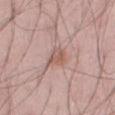Clinical impression:
Imaged during a routine full-body skin examination; the lesion was not biopsied and no histopathology is available.
Image and clinical context:
Measured at roughly 2.5 mm in maximum diameter. A male patient, about 50 years old. Captured under white-light illumination. Cropped from a total-body skin-imaging series; the visible field is about 15 mm. From the abdomen.